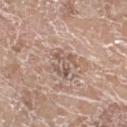The lesion was photographed on a routine skin check and not biopsied; there is no pathology result.
About 3 mm across.
The tile uses white-light illumination.
A 15 mm close-up tile from a total-body photography series done for melanoma screening.
The lesion is on the left lower leg.
A female patient aged around 75.